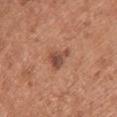Assessment: No biopsy was performed on this lesion — it was imaged during a full skin examination and was not determined to be concerning. Context: A female patient, aged 63 to 67. This is a white-light tile. From the chest. A region of skin cropped from a whole-body photographic capture, roughly 15 mm wide.